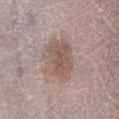<lesion>
<biopsy_status>not biopsied; imaged during a skin examination</biopsy_status>
<patient>
  <sex>female</sex>
  <age_approx>70</age_approx>
</patient>
<site>left lower leg</site>
<image>
  <source>total-body photography crop</source>
  <field_of_view_mm>15</field_of_view_mm>
</image>
</lesion>